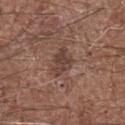Captured during whole-body skin photography for melanoma surveillance; the lesion was not biopsied.
A 15 mm crop from a total-body photograph taken for skin-cancer surveillance.
From the left forearm.
A male patient, roughly 70 years of age.
Captured under white-light illumination.
The total-body-photography lesion software estimated a border-irregularity rating of about 4/10 and a color-variation rating of about 2.5/10. The software also gave an automated nevus-likeness rating near 10 out of 100.